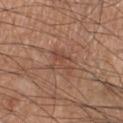Recorded during total-body skin imaging; not selected for excision or biopsy. A male patient, aged approximately 55. Captured under white-light illumination. Measured at roughly 3.5 mm in maximum diameter. The lesion is located on the right lower leg. This image is a 15 mm lesion crop taken from a total-body photograph.